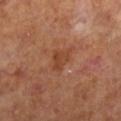Case summary:
– notes — total-body-photography surveillance lesion; no biopsy
– body site — the right lower leg
– lighting — cross-polarized
– subject — female, aged around 65
– acquisition — 15 mm crop, total-body photography